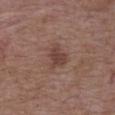follow-up: total-body-photography surveillance lesion; no biopsy
lighting: white-light
image-analysis metrics: a border-irregularity rating of about 3.5/10, a within-lesion color-variation index near 2/10, and a peripheral color-asymmetry measure near 0.5; a nevus-likeness score of about 35/100 and a lesion-detection confidence of about 100/100
patient: male, aged 48 to 52
imaging modality: ~15 mm tile from a whole-body skin photo
anatomic site: the upper back